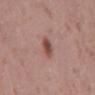Notes:
– image source: total-body-photography crop, ~15 mm field of view
– lesion size: ~3 mm (longest diameter)
– location: the mid back
– subject: male, in their mid-50s
– tile lighting: white-light